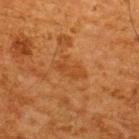Measured at roughly 4 mm in maximum diameter. An algorithmic analysis of the crop reported a lesion area of about 6 mm², an outline eccentricity of about 0.9 (0 = round, 1 = elongated), and two-axis asymmetry of about 0.25. The software also gave an average lesion color of about L≈38 a*≈23 b*≈36 (CIELAB) and about 5 CIELAB-L* units darker than the surrounding skin. And it measured border irregularity of about 4 on a 0–10 scale, internal color variation of about 2 on a 0–10 scale, and a peripheral color-asymmetry measure near 0.5. Imaged with cross-polarized lighting. The subject is a male aged 63–67. The lesion is on the upper back. Cropped from a total-body skin-imaging series; the visible field is about 15 mm.